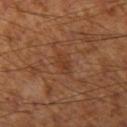Q: What is the imaging modality?
A: 15 mm crop, total-body photography
Q: What is the lesion's diameter?
A: ~3 mm (longest diameter)
Q: Lesion location?
A: the leg
Q: Who is the patient?
A: male, in their mid-50s
Q: Automated lesion metrics?
A: a footprint of about 3.5 mm² and a shape eccentricity near 0.85; a mean CIELAB color near L≈38 a*≈23 b*≈32, a lesion–skin lightness drop of about 6, and a normalized lesion–skin contrast near 5